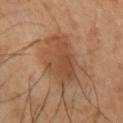Findings:
– notes · catalogued during a skin exam; not biopsied
– lesion diameter · ~6.5 mm (longest diameter)
– patient · female, about 45 years old
– lighting · cross-polarized illumination
– imaging modality · 15 mm crop, total-body photography
– body site · the left upper arm